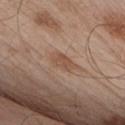biopsy_status: not biopsied; imaged during a skin examination
patient:
  sex: male
  age_approx: 55
lesion_size:
  long_diameter_mm_approx: 3.0
site: upper back
lighting: white-light
image:
  source: total-body photography crop
  field_of_view_mm: 15